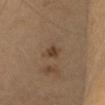automated_metrics:
  area_mm2_approx: 3.5
  eccentricity: 0.55
  shape_asymmetry: 0.25
  cielab_L: 37
  cielab_a: 15
  cielab_b: 26
  vs_skin_darker_L: 8.0
  vs_skin_contrast_norm: 7.5
image:
  source: total-body photography crop
  field_of_view_mm: 15
site: head or neck
patient:
  sex: female
  age_approx: 30
lighting: cross-polarized
lesion_size:
  long_diameter_mm_approx: 2.0